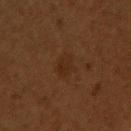Assessment: Captured during whole-body skin photography for melanoma surveillance; the lesion was not biopsied. Image and clinical context: About 3.5 mm across. The subject is a male aged 48–52. A lesion tile, about 15 mm wide, cut from a 3D total-body photograph. The lesion is on the chest.